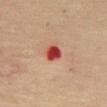The lesion was photographed on a routine skin check and not biopsied; there is no pathology result. The lesion is located on the abdomen. A female patient aged around 70. This is a cross-polarized tile. A close-up tile cropped from a whole-body skin photograph, about 15 mm across.